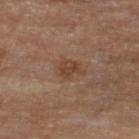Q: Was this lesion biopsied?
A: imaged on a skin check; not biopsied
Q: What is the lesion's diameter?
A: ≈3 mm
Q: What lighting was used for the tile?
A: cross-polarized illumination
Q: What is the anatomic site?
A: the leg
Q: What are the patient's age and sex?
A: male, aged approximately 85 or older
Q: What is the imaging modality?
A: ~15 mm tile from a whole-body skin photo